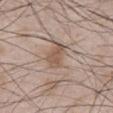{
  "biopsy_status": "not biopsied; imaged during a skin examination",
  "lesion_size": {
    "long_diameter_mm_approx": 3.0
  },
  "lighting": "white-light",
  "automated_metrics": {
    "vs_skin_darker_L": 9.0,
    "border_irregularity_0_10": 3.5,
    "peripheral_color_asymmetry": 1.0,
    "nevus_likeness_0_100": 80,
    "lesion_detection_confidence_0_100": 100
  },
  "site": "abdomen",
  "patient": {
    "sex": "male",
    "age_approx": 50
  },
  "image": {
    "source": "total-body photography crop",
    "field_of_view_mm": 15
  }
}